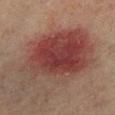follow-up: no biopsy performed (imaged during a skin exam)
tile lighting: cross-polarized illumination
location: the left lower leg
subject: male, about 75 years old
image source: 15 mm crop, total-body photography
image-analysis metrics: an eccentricity of roughly 0.65 and a symmetry-axis asymmetry near 0.25; a lesion color around L≈34 a*≈21 b*≈20 in CIELAB, roughly 9 lightness units darker than nearby skin, and a normalized border contrast of about 9; a border-irregularity index near 3.5/10 and a peripheral color-asymmetry measure near 2.5